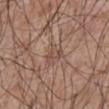The lesion was tiled from a total-body skin photograph and was not biopsied. Imaged with white-light lighting. The lesion-visualizer software estimated a footprint of about 4 mm² and an eccentricity of roughly 0.65. The analysis additionally found a lesion color around L≈49 a*≈18 b*≈25 in CIELAB, roughly 7 lightness units darker than nearby skin, and a normalized border contrast of about 5. The software also gave internal color variation of about 4 on a 0–10 scale and radial color variation of about 1.5. It also reported a classifier nevus-likeness of about 0/100 and lesion-presence confidence of about 75/100. A 15 mm close-up extracted from a 3D total-body photography capture. On the front of the torso. Longest diameter approximately 3 mm. The patient is a male aged 58 to 62.